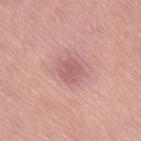{"biopsy_status": "not biopsied; imaged during a skin examination", "site": "abdomen", "image": {"source": "total-body photography crop", "field_of_view_mm": 15}, "lighting": "white-light", "lesion_size": {"long_diameter_mm_approx": 3.0}, "patient": {"sex": "female", "age_approx": 65}}